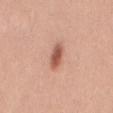Longest diameter approximately 3.5 mm. A 15 mm close-up extracted from a 3D total-body photography capture. The lesion is located on the abdomen. A female patient about 35 years old. The total-body-photography lesion software estimated a lesion area of about 5 mm² and a shape eccentricity near 0.9. The analysis additionally found a peripheral color-asymmetry measure near 1. The analysis additionally found lesion-presence confidence of about 100/100.